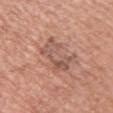biopsy_status: not biopsied; imaged during a skin examination
image:
  source: total-body photography crop
  field_of_view_mm: 15
site: right upper arm
lighting: white-light
lesion_size:
  long_diameter_mm_approx: 5.5
patient:
  sex: female
  age_approx: 60